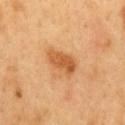Recorded during total-body skin imaging; not selected for excision or biopsy. The lesion is located on the back. A roughly 15 mm field-of-view crop from a total-body skin photograph. The patient is a male in their 50s. Captured under cross-polarized illumination.